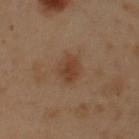<case>
<biopsy_status>not biopsied; imaged during a skin examination</biopsy_status>
<patient>
  <sex>male</sex>
  <age_approx>50</age_approx>
</patient>
<site>chest</site>
<lighting>cross-polarized</lighting>
<automated_metrics>
  <cielab_L>40</cielab_L>
  <cielab_a>18</cielab_a>
  <cielab_b>30</cielab_b>
  <border_irregularity_0_10>2.5</border_irregularity_0_10>
  <color_variation_0_10>2.0</color_variation_0_10>
  <peripheral_color_asymmetry>0.5</peripheral_color_asymmetry>
  <nevus_likeness_0_100>40</nevus_likeness_0_100>
  <lesion_detection_confidence_0_100>100</lesion_detection_confidence_0_100>
</automated_metrics>
<lesion_size>
  <long_diameter_mm_approx>3.5</long_diameter_mm_approx>
</lesion_size>
<image>
  <source>total-body photography crop</source>
  <field_of_view_mm>15</field_of_view_mm>
</image>
</case>